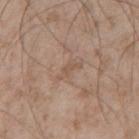| key | value |
|---|---|
| follow-up | total-body-photography surveillance lesion; no biopsy |
| anatomic site | the chest |
| image-analysis metrics | a mean CIELAB color near L≈54 a*≈16 b*≈28, a lesion–skin lightness drop of about 6, and a lesion-to-skin contrast of about 5 (normalized; higher = more distinct); a border-irregularity rating of about 5.5/10 and internal color variation of about 0 on a 0–10 scale; an automated nevus-likeness rating near 0 out of 100 |
| subject | male, aged 53 to 57 |
| imaging modality | ~15 mm tile from a whole-body skin photo |
| lighting | white-light illumination |
| size | about 3 mm |